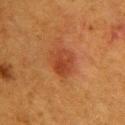workup=no biopsy performed (imaged during a skin exam); patient=female, approximately 40 years of age; image=~15 mm crop, total-body skin-cancer survey; anatomic site=the upper back.